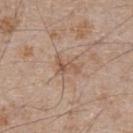{"biopsy_status": "not biopsied; imaged during a skin examination", "patient": {"sex": "male", "age_approx": 65}, "image": {"source": "total-body photography crop", "field_of_view_mm": 15}, "lighting": "white-light", "site": "chest", "automated_metrics": {"eccentricity": 0.8, "cielab_L": 55, "cielab_a": 18, "cielab_b": 29, "vs_skin_darker_L": 8.0, "vs_skin_contrast_norm": 5.5, "border_irregularity_0_10": 5.5, "color_variation_0_10": 4.5, "peripheral_color_asymmetry": 2.0, "nevus_likeness_0_100": 0, "lesion_detection_confidence_0_100": 100}}